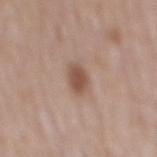Clinical impression: The lesion was tiled from a total-body skin photograph and was not biopsied.